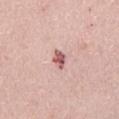No biopsy was performed on this lesion — it was imaged during a full skin examination and was not determined to be concerning. This image is a 15 mm lesion crop taken from a total-body photograph. The lesion's longest dimension is about 2.5 mm. A female patient, approximately 65 years of age. This is a white-light tile. On the front of the torso.